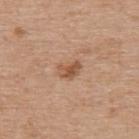| feature | finding |
|---|---|
| workup | no biopsy performed (imaged during a skin exam) |
| subject | female, roughly 65 years of age |
| acquisition | ~15 mm tile from a whole-body skin photo |
| location | the upper back |